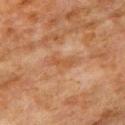– workup: imaged on a skin check; not biopsied
– anatomic site: the left upper arm
– imaging modality: ~15 mm crop, total-body skin-cancer survey
– subject: male, aged 78–82
– size: about 2.5 mm
– lighting: cross-polarized illumination
– automated metrics: a shape eccentricity near 0.95; an average lesion color of about L≈41 a*≈20 b*≈31 (CIELAB) and a lesion-to-skin contrast of about 6 (normalized; higher = more distinct); a border-irregularity index near 5/10, a color-variation rating of about 0/10, and peripheral color asymmetry of about 0; a nevus-likeness score of about 0/100 and a lesion-detection confidence of about 100/100